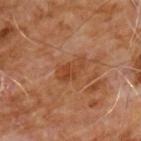Recorded during total-body skin imaging; not selected for excision or biopsy. The patient is a male approximately 60 years of age. A 15 mm close-up extracted from a 3D total-body photography capture. From the chest. Imaged with cross-polarized lighting. Approximately 4 mm at its widest.